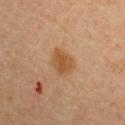Findings:
– biopsy status · no biopsy performed (imaged during a skin exam)
– tile lighting · cross-polarized illumination
– size · ~3.5 mm (longest diameter)
– image source · ~15 mm crop, total-body skin-cancer survey
– automated metrics · a color-variation rating of about 2/10 and radial color variation of about 0.5; an automated nevus-likeness rating near 85 out of 100 and a detector confidence of about 100 out of 100 that the crop contains a lesion
– location · the upper back
– subject · female, approximately 45 years of age